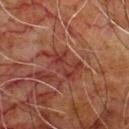Captured during whole-body skin photography for melanoma surveillance; the lesion was not biopsied. A male subject, aged around 80. Captured under cross-polarized illumination. On the front of the torso. About 5 mm across. A close-up tile cropped from a whole-body skin photograph, about 15 mm across.